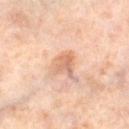This lesion was catalogued during total-body skin photography and was not selected for biopsy.
Measured at roughly 3.5 mm in maximum diameter.
On the right thigh.
The tile uses cross-polarized illumination.
Cropped from a total-body skin-imaging series; the visible field is about 15 mm.
A female patient roughly 50 years of age.